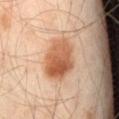Notes:
• biopsy status — catalogued during a skin exam; not biopsied
• image source — ~15 mm crop, total-body skin-cancer survey
• location — the right thigh
• lighting — cross-polarized illumination
• lesion diameter — ~5 mm (longest diameter)
• patient — male, aged approximately 45
• image-analysis metrics — a footprint of about 17 mm², a shape eccentricity near 0.6, and a shape-asymmetry score of about 0.1 (0 = symmetric); an average lesion color of about L≈57 a*≈23 b*≈35 (CIELAB), a lesion–skin lightness drop of about 13, and a normalized border contrast of about 9